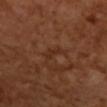Impression:
The lesion was tiled from a total-body skin photograph and was not biopsied.
Context:
Automated tile analysis of the lesion measured a lesion–skin lightness drop of about 5 and a normalized lesion–skin contrast near 5.5. The analysis additionally found border irregularity of about 5 on a 0–10 scale and a color-variation rating of about 0/10. A female patient, about 50 years old. About 3 mm across. The lesion is on the right forearm. A close-up tile cropped from a whole-body skin photograph, about 15 mm across. This is a cross-polarized tile.